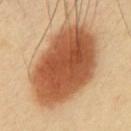Case summary:
• notes — total-body-photography surveillance lesion; no biopsy
• lesion size — ~10.5 mm (longest diameter)
• subject — male, aged 38–42
• lighting — cross-polarized illumination
• acquisition — 15 mm crop, total-body photography
• location — the front of the torso
• image-analysis metrics — a footprint of about 50 mm², an eccentricity of roughly 0.8, and a shape-asymmetry score of about 0.15 (0 = symmetric); a lesion color around L≈53 a*≈25 b*≈37 in CIELAB and a lesion–skin lightness drop of about 19